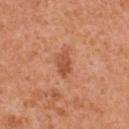Recorded during total-body skin imaging; not selected for excision or biopsy.